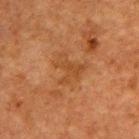Impression:
Imaged during a routine full-body skin examination; the lesion was not biopsied and no histopathology is available.
Context:
Cropped from a total-body skin-imaging series; the visible field is about 15 mm. A male subject, about 50 years old. The lesion is on the upper back.